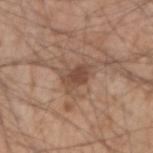This lesion was catalogued during total-body skin photography and was not selected for biopsy. A male subject aged 53 to 57. The lesion is on the right forearm. About 3.5 mm across. Imaged with white-light lighting. A roughly 15 mm field-of-view crop from a total-body skin photograph.